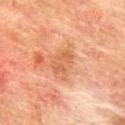Part of a total-body skin-imaging series; this lesion was reviewed on a skin check and was not flagged for biopsy.
A male patient aged 73–77.
The recorded lesion diameter is about 3.5 mm.
Cropped from a whole-body photographic skin survey; the tile spans about 15 mm.
The lesion is on the back.
The total-body-photography lesion software estimated an automated nevus-likeness rating near 0 out of 100 and lesion-presence confidence of about 100/100.